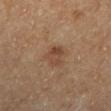The lesion was photographed on a routine skin check and not biopsied; there is no pathology result. An algorithmic analysis of the crop reported a lesion-detection confidence of about 100/100. The lesion is located on the left lower leg. Approximately 3 mm at its widest. A lesion tile, about 15 mm wide, cut from a 3D total-body photograph. The subject is a female aged approximately 60.